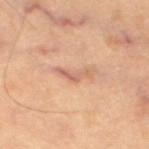Q: Is there a histopathology result?
A: catalogued during a skin exam; not biopsied
Q: Who is the patient?
A: male, aged approximately 60
Q: What kind of image is this?
A: ~15 mm tile from a whole-body skin photo
Q: What lighting was used for the tile?
A: cross-polarized
Q: Automated lesion metrics?
A: a border-irregularity rating of about 8.5/10 and radial color variation of about 0; a detector confidence of about 80 out of 100 that the crop contains a lesion
Q: How large is the lesion?
A: ~4 mm (longest diameter)
Q: Where on the body is the lesion?
A: the left thigh